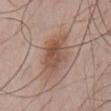The lesion was photographed on a routine skin check and not biopsied; there is no pathology result. The total-body-photography lesion software estimated a classifier nevus-likeness of about 90/100 and lesion-presence confidence of about 100/100. Captured under white-light illumination. A region of skin cropped from a whole-body photographic capture, roughly 15 mm wide. Measured at roughly 5 mm in maximum diameter. The lesion is on the chest. The subject is a male aged approximately 65.